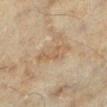Case summary:
* size · about 4 mm
* patient · female, roughly 60 years of age
* body site · the leg
* imaging modality · ~15 mm tile from a whole-body skin photo